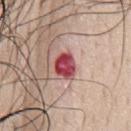Imaged during a routine full-body skin examination; the lesion was not biopsied and no histopathology is available.
Cropped from a whole-body photographic skin survey; the tile spans about 15 mm.
Located on the chest.
A male patient, in their 70s.
The tile uses white-light illumination.
Measured at roughly 3.5 mm in maximum diameter.